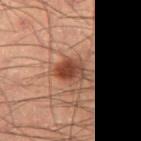Notes:
– notes · catalogued during a skin exam; not biopsied
– site · the leg
– automated lesion analysis · a border-irregularity index near 2/10 and a color-variation rating of about 4/10; a classifier nevus-likeness of about 95/100 and a detector confidence of about 100 out of 100 that the crop contains a lesion
– illumination · cross-polarized
– patient · male, roughly 55 years of age
– image · ~15 mm tile from a whole-body skin photo
– diameter · about 3.5 mm